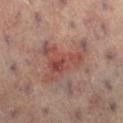Assessment: This lesion was catalogued during total-body skin photography and was not selected for biopsy. Context: A 15 mm close-up extracted from a 3D total-body photography capture. The patient is a female approximately 80 years of age. From the left leg. About 5.5 mm across.